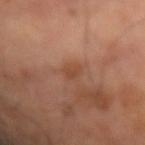The lesion was photographed on a routine skin check and not biopsied; there is no pathology result. Longest diameter approximately 2.5 mm. Captured under cross-polarized illumination. The lesion is located on the left forearm. Cropped from a whole-body photographic skin survey; the tile spans about 15 mm. The lesion-visualizer software estimated a footprint of about 3 mm², an outline eccentricity of about 0.75 (0 = round, 1 = elongated), and two-axis asymmetry of about 0.3. The software also gave a border-irregularity index near 3/10, a within-lesion color-variation index near 1.5/10, and peripheral color asymmetry of about 0.5. The analysis additionally found a lesion-detection confidence of about 100/100. The subject is a male in their mid- to late 60s.